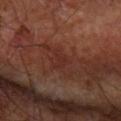Captured during whole-body skin photography for melanoma surveillance; the lesion was not biopsied. The lesion-visualizer software estimated a symmetry-axis asymmetry near 0.55. It also reported a lesion color around L≈28 a*≈23 b*≈23 in CIELAB, about 4 CIELAB-L* units darker than the surrounding skin, and a normalized lesion–skin contrast near 4.5. It also reported a border-irregularity index near 5.5/10, a color-variation rating of about 0/10, and radial color variation of about 0. The analysis additionally found a nevus-likeness score of about 0/100 and lesion-presence confidence of about 95/100. Measured at roughly 2.5 mm in maximum diameter. A male patient in their 60s. Imaged with cross-polarized lighting. From the right forearm. Cropped from a total-body skin-imaging series; the visible field is about 15 mm.